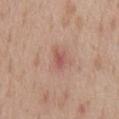Findings:
* follow-up · no biopsy performed (imaged during a skin exam)
* image · ~15 mm tile from a whole-body skin photo
* size · about 2.5 mm
* location · the chest
* subject · male, aged approximately 55
* illumination · white-light